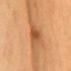Q: Was a biopsy performed?
A: total-body-photography surveillance lesion; no biopsy
Q: Illumination type?
A: cross-polarized illumination
Q: Lesion location?
A: the mid back
Q: Automated lesion metrics?
A: an automated nevus-likeness rating near 0 out of 100
Q: What are the patient's age and sex?
A: male, in their 60s
Q: What kind of image is this?
A: 15 mm crop, total-body photography
Q: What is the lesion's diameter?
A: ~4 mm (longest diameter)